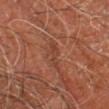Assessment:
The lesion was tiled from a total-body skin photograph and was not biopsied.
Image and clinical context:
Automated tile analysis of the lesion measured a lesion color around L≈40 a*≈24 b*≈29 in CIELAB. It also reported a border-irregularity rating of about 4.5/10, a within-lesion color-variation index near 0.5/10, and radial color variation of about 0. The lesion is on the right leg. A lesion tile, about 15 mm wide, cut from a 3D total-body photograph. A male subject, roughly 60 years of age. This is a cross-polarized tile. About 3.5 mm across.